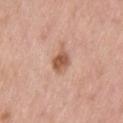workup: no biopsy performed (imaged during a skin exam) | image source: ~15 mm crop, total-body skin-cancer survey | anatomic site: the back | patient: male, in their mid- to late 40s.